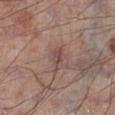Clinical impression:
Part of a total-body skin-imaging series; this lesion was reviewed on a skin check and was not flagged for biopsy.
Image and clinical context:
This is a white-light tile. A 15 mm close-up tile from a total-body photography series done for melanoma screening. Longest diameter approximately 3 mm. Located on the right lower leg. A male subject, about 70 years old.